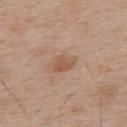A roughly 15 mm field-of-view crop from a total-body skin photograph. The lesion is located on the upper back. The lesion's longest dimension is about 3 mm. A male patient aged around 70. An algorithmic analysis of the crop reported an area of roughly 4.5 mm², an eccentricity of roughly 0.8, and two-axis asymmetry of about 0.35. It also reported a mean CIELAB color near L≈54 a*≈19 b*≈30. And it measured border irregularity of about 3 on a 0–10 scale, a color-variation rating of about 1.5/10, and peripheral color asymmetry of about 0.5. This is a white-light tile.